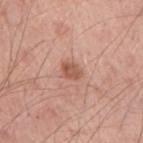biopsy_status: not biopsied; imaged during a skin examination
site: right upper arm
lighting: white-light
patient:
  sex: male
  age_approx: 60
lesion_size:
  long_diameter_mm_approx: 2.5
image:
  source: total-body photography crop
  field_of_view_mm: 15
automated_metrics:
  border_irregularity_0_10: 2.5
  color_variation_0_10: 2.5
  peripheral_color_asymmetry: 1.0
  nevus_likeness_0_100: 75
  lesion_detection_confidence_0_100: 100